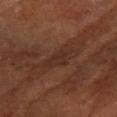body site=the left arm
imaging modality=~15 mm tile from a whole-body skin photo
patient=female, in their mid-60s